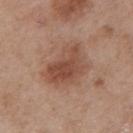The lesion was tiled from a total-body skin photograph and was not biopsied. A roughly 15 mm field-of-view crop from a total-body skin photograph. The patient is a male aged approximately 55. An algorithmic analysis of the crop reported a footprint of about 16 mm², a shape eccentricity near 0.7, and a symmetry-axis asymmetry near 0.3. The software also gave an average lesion color of about L≈49 a*≈21 b*≈29 (CIELAB), a lesion–skin lightness drop of about 10, and a normalized border contrast of about 7.5. The analysis additionally found an automated nevus-likeness rating near 20 out of 100 and a detector confidence of about 100 out of 100 that the crop contains a lesion. The lesion is located on the upper back. Imaged with white-light lighting. Measured at roughly 5.5 mm in maximum diameter.